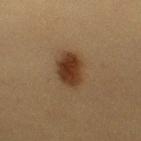• biopsy status · catalogued during a skin exam; not biopsied
• body site · the right upper arm
• acquisition · ~15 mm crop, total-body skin-cancer survey
• size · ≈4 mm
• subject · male, roughly 35 years of age
• TBP lesion metrics · two-axis asymmetry of about 0.15; about 12 CIELAB-L* units darker than the surrounding skin and a normalized lesion–skin contrast near 11.5; a within-lesion color-variation index near 3.5/10 and peripheral color asymmetry of about 1; a classifier nevus-likeness of about 100/100